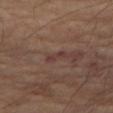biopsy status: imaged on a skin check; not biopsied | anatomic site: the leg | illumination: cross-polarized illumination | image source: ~15 mm crop, total-body skin-cancer survey | TBP lesion metrics: internal color variation of about 0 on a 0–10 scale and radial color variation of about 0; a nevus-likeness score of about 0/100 and lesion-presence confidence of about 75/100 | patient: male, approximately 70 years of age.